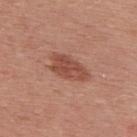Case summary:
• notes — no biopsy performed (imaged during a skin exam)
• acquisition — ~15 mm crop, total-body skin-cancer survey
• subject — male, about 30 years old
• body site — the upper back